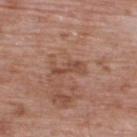Recorded during total-body skin imaging; not selected for excision or biopsy. An algorithmic analysis of the crop reported a lesion area of about 3.5 mm², an outline eccentricity of about 0.95 (0 = round, 1 = elongated), and two-axis asymmetry of about 0.4. And it measured a lesion color around L≈47 a*≈22 b*≈29 in CIELAB, a lesion–skin lightness drop of about 8, and a normalized lesion–skin contrast near 6.5. Located on the upper back. This image is a 15 mm lesion crop taken from a total-body photograph. Imaged with white-light lighting. Measured at roughly 3.5 mm in maximum diameter. A male subject, roughly 70 years of age.